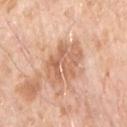Q: Was this lesion biopsied?
A: total-body-photography surveillance lesion; no biopsy
Q: Lesion location?
A: the chest
Q: Patient demographics?
A: male, aged 68 to 72
Q: Lesion size?
A: ≈5.5 mm
Q: How was the tile lit?
A: white-light
Q: What kind of image is this?
A: ~15 mm crop, total-body skin-cancer survey